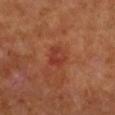Findings:
• follow-up: catalogued during a skin exam; not biopsied
• size: ~3 mm (longest diameter)
• image source: ~15 mm tile from a whole-body skin photo
• illumination: cross-polarized illumination
• body site: the right forearm
• subject: male, about 55 years old